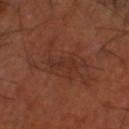Part of a total-body skin-imaging series; this lesion was reviewed on a skin check and was not flagged for biopsy.
A male subject, about 60 years old.
The lesion's longest dimension is about 4.5 mm.
The lesion is located on the head or neck.
The lesion-visualizer software estimated a nevus-likeness score of about 0/100 and a detector confidence of about 90 out of 100 that the crop contains a lesion.
The tile uses cross-polarized illumination.
A 15 mm close-up extracted from a 3D total-body photography capture.